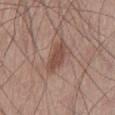<case>
<biopsy_status>not biopsied; imaged during a skin examination</biopsy_status>
<site>left thigh</site>
<image>
  <source>total-body photography crop</source>
  <field_of_view_mm>15</field_of_view_mm>
</image>
<lighting>white-light</lighting>
<patient>
  <sex>male</sex>
  <age_approx>55</age_approx>
</patient>
<lesion_size>
  <long_diameter_mm_approx>5.0</long_diameter_mm_approx>
</lesion_size>
</case>